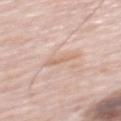| key | value |
|---|---|
| notes | imaged on a skin check; not biopsied |
| body site | the mid back |
| subject | male, aged approximately 80 |
| image | ~15 mm crop, total-body skin-cancer survey |